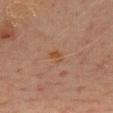No biopsy was performed on this lesion — it was imaged during a full skin examination and was not determined to be concerning. A lesion tile, about 15 mm wide, cut from a 3D total-body photograph. Located on the chest. The total-body-photography lesion software estimated a mean CIELAB color near L≈40 a*≈17 b*≈28 and a lesion-to-skin contrast of about 6.5 (normalized; higher = more distinct). It also reported a lesion-detection confidence of about 100/100. A male subject approximately 70 years of age.